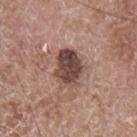{
  "biopsy_status": "not biopsied; imaged during a skin examination",
  "patient": {
    "sex": "male",
    "age_approx": 60
  },
  "lesion_size": {
    "long_diameter_mm_approx": 4.5
  },
  "lighting": "white-light",
  "site": "left forearm",
  "image": {
    "source": "total-body photography crop",
    "field_of_view_mm": 15
  }
}